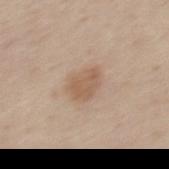Clinical impression: Recorded during total-body skin imaging; not selected for excision or biopsy. Image and clinical context: Imaged with white-light lighting. The patient is a male approximately 45 years of age. Approximately 3.5 mm at its widest. A 15 mm crop from a total-body photograph taken for skin-cancer surveillance. From the mid back.